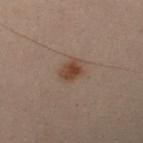Imaged during a routine full-body skin examination; the lesion was not biopsied and no histopathology is available. A roughly 15 mm field-of-view crop from a total-body skin photograph. Automated image analysis of the tile measured an area of roughly 5 mm² and an outline eccentricity of about 0.6 (0 = round, 1 = elongated). The software also gave a border-irregularity index near 2.5/10 and radial color variation of about 1. It also reported a lesion-detection confidence of about 100/100. From the left forearm. A male subject, roughly 50 years of age. Captured under cross-polarized illumination.